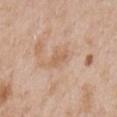Imaged during a routine full-body skin examination; the lesion was not biopsied and no histopathology is available.
The lesion's longest dimension is about 3 mm.
Captured under white-light illumination.
Located on the chest.
The subject is a male about 65 years old.
A roughly 15 mm field-of-view crop from a total-body skin photograph.